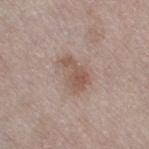workup: total-body-photography surveillance lesion; no biopsy
location: the right thigh
lesion diameter: ≈4 mm
patient: female, roughly 60 years of age
image: 15 mm crop, total-body photography
automated lesion analysis: a nevus-likeness score of about 40/100 and a lesion-detection confidence of about 100/100
lighting: white-light illumination